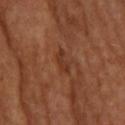{"biopsy_status": "not biopsied; imaged during a skin examination", "image": {"source": "total-body photography crop", "field_of_view_mm": 15}, "site": "head or neck", "lesion_size": {"long_diameter_mm_approx": 3.0}, "lighting": "cross-polarized"}